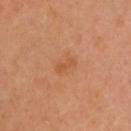Captured during whole-body skin photography for melanoma surveillance; the lesion was not biopsied. The lesion's longest dimension is about 3 mm. A close-up tile cropped from a whole-body skin photograph, about 15 mm across. On the head or neck. This is a cross-polarized tile. A female patient roughly 40 years of age. The total-body-photography lesion software estimated a nevus-likeness score of about 0/100 and a detector confidence of about 100 out of 100 that the crop contains a lesion.